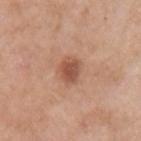Captured during whole-body skin photography for melanoma surveillance; the lesion was not biopsied. A female patient aged 53 to 57. The total-body-photography lesion software estimated a border-irregularity index near 1.5/10 and a color-variation rating of about 3/10. Approximately 3 mm at its widest. Imaged with white-light lighting. Located on the left upper arm. A roughly 15 mm field-of-view crop from a total-body skin photograph.